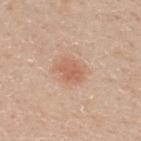Q: Was this lesion biopsied?
A: catalogued during a skin exam; not biopsied
Q: Automated lesion metrics?
A: a lesion area of about 7 mm² and two-axis asymmetry of about 0.25; a lesion color around L≈61 a*≈22 b*≈31 in CIELAB, a lesion–skin lightness drop of about 8, and a lesion-to-skin contrast of about 5.5 (normalized; higher = more distinct); border irregularity of about 2.5 on a 0–10 scale, a color-variation rating of about 2/10, and peripheral color asymmetry of about 0.5; a classifier nevus-likeness of about 85/100
Q: Lesion size?
A: ~3.5 mm (longest diameter)
Q: Patient demographics?
A: male, aged approximately 30
Q: How was the tile lit?
A: white-light
Q: What is the imaging modality?
A: 15 mm crop, total-body photography
Q: What is the anatomic site?
A: the upper back